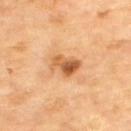The lesion was photographed on a routine skin check and not biopsied; there is no pathology result.
Captured under cross-polarized illumination.
A roughly 15 mm field-of-view crop from a total-body skin photograph.
The lesion is on the upper back.
The lesion's longest dimension is about 3.5 mm.
A male patient aged 68 to 72.
The total-body-photography lesion software estimated a lesion color around L≈59 a*≈25 b*≈41 in CIELAB, about 13 CIELAB-L* units darker than the surrounding skin, and a lesion-to-skin contrast of about 8.5 (normalized; higher = more distinct). It also reported a border-irregularity index near 3.5/10, a within-lesion color-variation index near 9/10, and radial color variation of about 3. And it measured an automated nevus-likeness rating near 55 out of 100 and lesion-presence confidence of about 100/100.